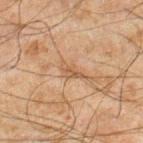Findings:
- follow-up — total-body-photography surveillance lesion; no biopsy
- subject — male, about 45 years old
- body site — the right lower leg
- lesion size — ~2.5 mm (longest diameter)
- illumination — cross-polarized
- imaging modality — total-body-photography crop, ~15 mm field of view